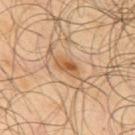Notes:
– workup: no biopsy performed (imaged during a skin exam)
– image source: ~15 mm tile from a whole-body skin photo
– subject: male, approximately 65 years of age
– site: the upper back
– automated metrics: an area of roughly 4.5 mm², an eccentricity of roughly 0.9, and two-axis asymmetry of about 0.3; roughly 10 lightness units darker than nearby skin; a classifier nevus-likeness of about 75/100 and lesion-presence confidence of about 100/100